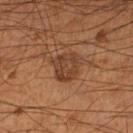| field | value |
|---|---|
| follow-up | catalogued during a skin exam; not biopsied |
| automated metrics | an area of roughly 9 mm², a shape eccentricity near 0.3, and a symmetry-axis asymmetry near 0.3; a mean CIELAB color near L≈37 a*≈19 b*≈29, about 8 CIELAB-L* units darker than the surrounding skin, and a normalized border contrast of about 7; a nevus-likeness score of about 30/100 and a detector confidence of about 100 out of 100 that the crop contains a lesion |
| image | 15 mm crop, total-body photography |
| location | the left lower leg |
| illumination | cross-polarized |
| patient | male, approximately 55 years of age |